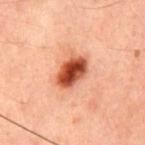– follow-up: imaged on a skin check; not biopsied
– automated metrics: a footprint of about 9 mm², a shape eccentricity near 0.7, and a symmetry-axis asymmetry near 0.15; about 17 CIELAB-L* units darker than the surrounding skin and a lesion-to-skin contrast of about 13 (normalized; higher = more distinct); lesion-presence confidence of about 100/100
– anatomic site: the front of the torso
– subject: male, in their 60s
– illumination: cross-polarized illumination
– image source: ~15 mm tile from a whole-body skin photo